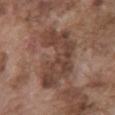Captured during whole-body skin photography for melanoma surveillance; the lesion was not biopsied. The tile uses white-light illumination. About 8 mm across. The lesion is on the front of the torso. A male patient about 75 years old. A 15 mm close-up tile from a total-body photography series done for melanoma screening.